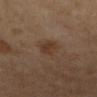Clinical impression:
Captured during whole-body skin photography for melanoma surveillance; the lesion was not biopsied.
Image and clinical context:
Imaged with cross-polarized lighting. From the leg. The total-body-photography lesion software estimated a border-irregularity index near 2.5/10, a color-variation rating of about 2/10, and radial color variation of about 1. And it measured a classifier nevus-likeness of about 50/100 and lesion-presence confidence of about 100/100. Longest diameter approximately 3 mm. Cropped from a total-body skin-imaging series; the visible field is about 15 mm. The subject is a female aged approximately 60.